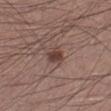Captured during whole-body skin photography for melanoma surveillance; the lesion was not biopsied.
From the left lower leg.
Automated image analysis of the tile measured an area of roughly 4.5 mm² and a symmetry-axis asymmetry near 0.3. It also reported a lesion–skin lightness drop of about 10 and a lesion-to-skin contrast of about 8.5 (normalized; higher = more distinct). And it measured a border-irregularity rating of about 2.5/10, a color-variation rating of about 3/10, and radial color variation of about 1.
A male patient in their mid-30s.
Cropped from a total-body skin-imaging series; the visible field is about 15 mm.
Approximately 2.5 mm at its widest.